A male patient roughly 70 years of age; a 15 mm close-up extracted from a 3D total-body photography capture; from the front of the torso; captured under cross-polarized illumination:
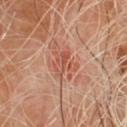Conclusion: The biopsy diagnosis was a malignancy: nodular basal cell carcinoma.